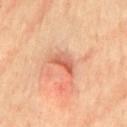Q: Was this lesion biopsied?
A: catalogued during a skin exam; not biopsied
Q: What are the patient's age and sex?
A: female, approximately 65 years of age
Q: What is the anatomic site?
A: the left thigh
Q: What lighting was used for the tile?
A: cross-polarized
Q: What is the lesion's diameter?
A: ~3.5 mm (longest diameter)
Q: What is the imaging modality?
A: 15 mm crop, total-body photography